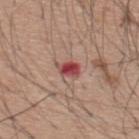• biopsy status · catalogued during a skin exam; not biopsied
• lighting · white-light illumination
• imaging modality · ~15 mm crop, total-body skin-cancer survey
• patient · male, aged around 60
• lesion size · about 3 mm
• location · the upper back